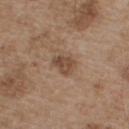site: the chest | tile lighting: white-light | patient: male, aged approximately 55 | lesion diameter: about 2.5 mm | automated metrics: a mean CIELAB color near L≈46 a*≈17 b*≈28, a lesion–skin lightness drop of about 10, and a lesion-to-skin contrast of about 7.5 (normalized; higher = more distinct); lesion-presence confidence of about 100/100 | imaging modality: total-body-photography crop, ~15 mm field of view.